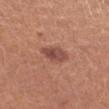No biopsy was performed on this lesion — it was imaged during a full skin examination and was not determined to be concerning.
The total-body-photography lesion software estimated a footprint of about 6.5 mm², an outline eccentricity of about 0.75 (0 = round, 1 = elongated), and a shape-asymmetry score of about 0.15 (0 = symmetric).
On the left forearm.
Cropped from a total-body skin-imaging series; the visible field is about 15 mm.
A female patient, roughly 25 years of age.